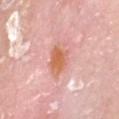Clinical impression:
Recorded during total-body skin imaging; not selected for excision or biopsy.
Image and clinical context:
A male subject aged 63 to 67. Cropped from a total-body skin-imaging series; the visible field is about 15 mm. Located on the head or neck. Captured under white-light illumination. Automated image analysis of the tile measured a border-irregularity rating of about 2.5/10 and a within-lesion color-variation index near 5.5/10. And it measured a nevus-likeness score of about 20/100 and lesion-presence confidence of about 100/100. Approximately 4 mm at its widest.